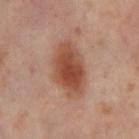{
  "biopsy_status": "not biopsied; imaged during a skin examination",
  "image": {
    "source": "total-body photography crop",
    "field_of_view_mm": 15
  },
  "site": "left thigh",
  "lesion_size": {
    "long_diameter_mm_approx": 6.0
  },
  "patient": {
    "sex": "female",
    "age_approx": 55
  },
  "lighting": "cross-polarized"
}